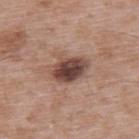The tile uses white-light illumination.
A male patient, aged around 50.
Located on the upper back.
A roughly 15 mm field-of-view crop from a total-body skin photograph.
The total-body-photography lesion software estimated an eccentricity of roughly 0.75 and a symmetry-axis asymmetry near 0.2. And it measured a lesion color around L≈45 a*≈19 b*≈23 in CIELAB and a normalized border contrast of about 11.5.